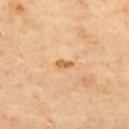{
  "biopsy_status": "not biopsied; imaged during a skin examination",
  "lighting": "cross-polarized",
  "automated_metrics": {
    "area_mm2_approx": 2.0,
    "eccentricity": 0.85,
    "shape_asymmetry": 0.35,
    "nevus_likeness_0_100": 20,
    "lesion_detection_confidence_0_100": 100
  },
  "patient": {
    "sex": "male",
    "age_approx": 65
  },
  "image": {
    "source": "total-body photography crop",
    "field_of_view_mm": 15
  },
  "site": "upper back"
}